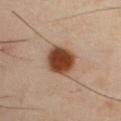Q: Is there a histopathology result?
A: catalogued during a skin exam; not biopsied
Q: Lesion location?
A: the chest
Q: Lesion size?
A: ≈4 mm
Q: Who is the patient?
A: male, in their 40s
Q: What did automated image analysis measure?
A: a lesion color around L≈42 a*≈22 b*≈31 in CIELAB and a lesion-to-skin contrast of about 13.5 (normalized; higher = more distinct)
Q: What kind of image is this?
A: 15 mm crop, total-body photography
Q: Illumination type?
A: cross-polarized illumination